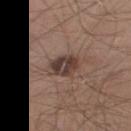lesion diameter = about 3.5 mm; image source = ~15 mm crop, total-body skin-cancer survey; subject = male, aged 28–32; lighting = white-light illumination; body site = the right lower leg.